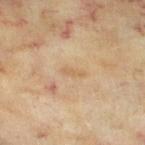<case>
<biopsy_status>not biopsied; imaged during a skin examination</biopsy_status>
<automated_metrics>
  <area_mm2_approx>2.0</area_mm2_approx>
  <eccentricity>0.95</eccentricity>
  <shape_asymmetry>0.4</shape_asymmetry>
  <cielab_L>57</cielab_L>
  <cielab_a>15</cielab_a>
  <cielab_b>34</cielab_b>
  <vs_skin_darker_L>6.0</vs_skin_darker_L>
  <vs_skin_contrast_norm>4.5</vs_skin_contrast_norm>
  <border_irregularity_0_10>4.5</border_irregularity_0_10>
  <color_variation_0_10>0.0</color_variation_0_10>
  <peripheral_color_asymmetry>0.0</peripheral_color_asymmetry>
  <nevus_likeness_0_100>0</nevus_likeness_0_100>
  <lesion_detection_confidence_0_100>100</lesion_detection_confidence_0_100>
</automated_metrics>
<patient>
  <sex>female</sex>
  <age_approx>60</age_approx>
</patient>
<image>
  <source>total-body photography crop</source>
  <field_of_view_mm>15</field_of_view_mm>
</image>
<lesion_size>
  <long_diameter_mm_approx>2.5</long_diameter_mm_approx>
</lesion_size>
<site>left thigh</site>
</case>